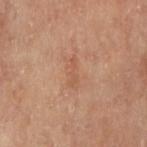Assessment: The lesion was tiled from a total-body skin photograph and was not biopsied.